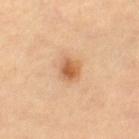Assessment:
Imaged during a routine full-body skin examination; the lesion was not biopsied and no histopathology is available.
Clinical summary:
Automated tile analysis of the lesion measured an area of roughly 5.5 mm² and a shape eccentricity near 0.55. The analysis additionally found a border-irregularity index near 2/10, a color-variation rating of about 4.5/10, and peripheral color asymmetry of about 1.5. And it measured an automated nevus-likeness rating near 95 out of 100. Imaged with cross-polarized lighting. A close-up tile cropped from a whole-body skin photograph, about 15 mm across. On the leg. About 3 mm across. A female patient, aged 68–72.